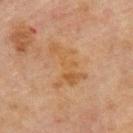notes: total-body-photography surveillance lesion; no biopsy | illumination: cross-polarized illumination | site: the upper back | subject: male, approximately 70 years of age | acquisition: total-body-photography crop, ~15 mm field of view.